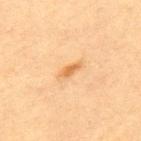Impression:
Recorded during total-body skin imaging; not selected for excision or biopsy.